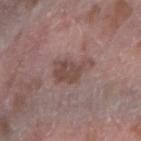Q: Was this lesion biopsied?
A: no biopsy performed (imaged during a skin exam)
Q: What is the imaging modality?
A: total-body-photography crop, ~15 mm field of view
Q: Patient demographics?
A: female, about 65 years old
Q: What is the lesion's diameter?
A: about 5 mm
Q: Lesion location?
A: the right forearm
Q: How was the tile lit?
A: white-light